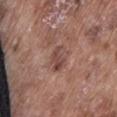biopsy status: total-body-photography surveillance lesion; no biopsy
patient: male, aged around 75
site: the abdomen
imaging modality: total-body-photography crop, ~15 mm field of view